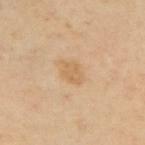Impression:
This lesion was catalogued during total-body skin photography and was not selected for biopsy.
Context:
Longest diameter approximately 2.5 mm. A male patient, about 65 years old. A close-up tile cropped from a whole-body skin photograph, about 15 mm across. On the upper back.